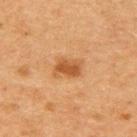Findings:
- workup: imaged on a skin check; not biopsied
- automated lesion analysis: an average lesion color of about L≈43 a*≈22 b*≈36 (CIELAB), roughly 10 lightness units darker than nearby skin, and a normalized border contrast of about 8
- tile lighting: cross-polarized
- body site: the back
- image: 15 mm crop, total-body photography
- patient: male, aged approximately 50
- lesion size: ≈3 mm